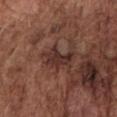A male subject, in their mid- to late 70s. An algorithmic analysis of the crop reported an area of roughly 7 mm², an eccentricity of roughly 0.8, and a symmetry-axis asymmetry near 0.35. Located on the chest. Cropped from a total-body skin-imaging series; the visible field is about 15 mm. The lesion's longest dimension is about 4 mm.